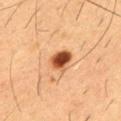Part of a total-body skin-imaging series; this lesion was reviewed on a skin check and was not flagged for biopsy.
A region of skin cropped from a whole-body photographic capture, roughly 15 mm wide.
The tile uses cross-polarized illumination.
Longest diameter approximately 3 mm.
The lesion is located on the chest.
A male patient in their mid-50s.
The lesion-visualizer software estimated a border-irregularity index near 2/10, internal color variation of about 6 on a 0–10 scale, and a peripheral color-asymmetry measure near 1.5. The software also gave a nevus-likeness score of about 100/100 and lesion-presence confidence of about 100/100.